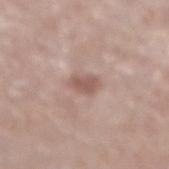This lesion was catalogued during total-body skin photography and was not selected for biopsy. The lesion is on the right lower leg. A female patient, in their mid-70s. A region of skin cropped from a whole-body photographic capture, roughly 15 mm wide.